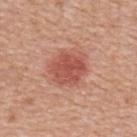Recorded during total-body skin imaging; not selected for excision or biopsy.
A female patient roughly 50 years of age.
A 15 mm close-up extracted from a 3D total-body photography capture.
The lesion is on the upper back.